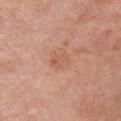Impression: Part of a total-body skin-imaging series; this lesion was reviewed on a skin check and was not flagged for biopsy. Image and clinical context: Automated image analysis of the tile measured border irregularity of about 3 on a 0–10 scale and radial color variation of about 1. About 2.5 mm across. A male subject in their mid- to late 50s. From the left upper arm. Imaged with white-light lighting. A close-up tile cropped from a whole-body skin photograph, about 15 mm across.